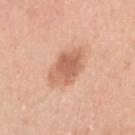Recorded during total-body skin imaging; not selected for excision or biopsy. A female patient approximately 40 years of age. The total-body-photography lesion software estimated an area of roughly 12 mm² and an eccentricity of roughly 0.65. It also reported a lesion color around L≈63 a*≈24 b*≈34 in CIELAB, a lesion–skin lightness drop of about 12, and a lesion-to-skin contrast of about 7.5 (normalized; higher = more distinct). The analysis additionally found a classifier nevus-likeness of about 75/100 and a detector confidence of about 100 out of 100 that the crop contains a lesion. Cropped from a total-body skin-imaging series; the visible field is about 15 mm. From the right upper arm.